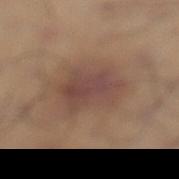notes: no biopsy performed (imaged during a skin exam)
automated lesion analysis: lesion-presence confidence of about 100/100
imaging modality: ~15 mm tile from a whole-body skin photo
anatomic site: the left lower leg
diameter: about 5.5 mm
patient: male, in their mid-50s
tile lighting: white-light